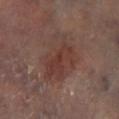– workup — imaged on a skin check; not biopsied
– anatomic site — the left lower leg
– illumination — cross-polarized illumination
– image — ~15 mm tile from a whole-body skin photo
– diameter — ~6.5 mm (longest diameter)
– TBP lesion metrics — a border-irregularity rating of about 3.5/10 and radial color variation of about 1.5
– subject — male, approximately 65 years of age